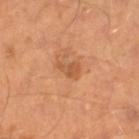Findings:
* workup — total-body-photography surveillance lesion; no biopsy
* imaging modality — total-body-photography crop, ~15 mm field of view
* illumination — cross-polarized illumination
* lesion size — ~3 mm (longest diameter)
* subject — male, aged 38–42
* automated lesion analysis — an eccentricity of roughly 0.8 and a symmetry-axis asymmetry near 0.45; a mean CIELAB color near L≈55 a*≈26 b*≈39 and roughly 8 lightness units darker than nearby skin; border irregularity of about 4.5 on a 0–10 scale
* location — the right lower leg